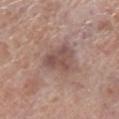| key | value |
|---|---|
| body site | the left lower leg |
| acquisition | ~15 mm tile from a whole-body skin photo |
| size | ≈4 mm |
| patient | male, in their 70s |
| illumination | white-light |